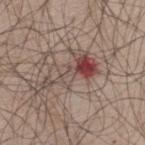Assessment: This lesion was catalogued during total-body skin photography and was not selected for biopsy. Acquisition and patient details: The lesion-visualizer software estimated a footprint of about 10 mm², an eccentricity of roughly 0.95, and two-axis asymmetry of about 0.65. It also reported roughly 11 lightness units darker than nearby skin and a normalized border contrast of about 8.5. It also reported a lesion-detection confidence of about 95/100. A region of skin cropped from a whole-body photographic capture, roughly 15 mm wide. Longest diameter approximately 7 mm. The lesion is on the upper back. Captured under white-light illumination. The subject is a male aged around 45.